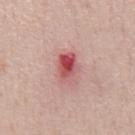Notes:
– biopsy status — total-body-photography surveillance lesion; no biopsy
– imaging modality — ~15 mm crop, total-body skin-cancer survey
– size — ≈4 mm
– subject — male, approximately 50 years of age
– body site — the abdomen
– lighting — white-light